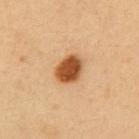Clinical summary:
A male subject about 50 years old. A 15 mm close-up tile from a total-body photography series done for melanoma screening. The lesion is on the abdomen. This is a cross-polarized tile. An algorithmic analysis of the crop reported a lesion area of about 8.5 mm², an eccentricity of roughly 0.6, and a symmetry-axis asymmetry near 0.1.